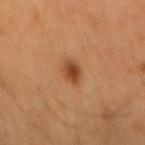This lesion was catalogued during total-body skin photography and was not selected for biopsy. From the back. A male patient roughly 65 years of age. Imaged with cross-polarized lighting. Automated image analysis of the tile measured a footprint of about 4.5 mm² and a shape eccentricity near 0.75. The analysis additionally found a classifier nevus-likeness of about 95/100 and a lesion-detection confidence of about 100/100. A 15 mm close-up tile from a total-body photography series done for melanoma screening.